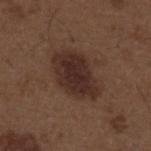Q: Is there a histopathology result?
A: total-body-photography surveillance lesion; no biopsy
Q: What is the anatomic site?
A: the upper back
Q: Patient demographics?
A: male, aged 48 to 52
Q: How was this image acquired?
A: total-body-photography crop, ~15 mm field of view
Q: Illumination type?
A: white-light
Q: Lesion size?
A: ~6 mm (longest diameter)